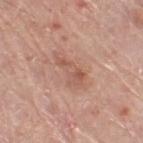Case summary:
– notes — catalogued during a skin exam; not biopsied
– image — total-body-photography crop, ~15 mm field of view
– location — the leg
– automated lesion analysis — an eccentricity of roughly 0.9; a nevus-likeness score of about 10/100 and a lesion-detection confidence of about 100/100
– subject — male, aged 78 to 82
– illumination — white-light illumination